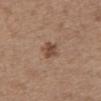Captured during whole-body skin photography for melanoma surveillance; the lesion was not biopsied.
A 15 mm close-up tile from a total-body photography series done for melanoma screening.
The lesion is on the abdomen.
Approximately 3 mm at its widest.
A female patient aged around 65.